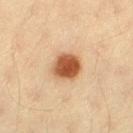Q: What kind of image is this?
A: total-body-photography crop, ~15 mm field of view
Q: Illumination type?
A: cross-polarized
Q: Who is the patient?
A: female, roughly 40 years of age
Q: What is the lesion's diameter?
A: about 3.5 mm
Q: Where on the body is the lesion?
A: the left thigh